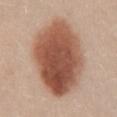Findings:
– follow-up: no biopsy performed (imaged during a skin exam)
– diameter: ~10 mm (longest diameter)
– image-analysis metrics: a symmetry-axis asymmetry near 0.1; a lesion–skin lightness drop of about 17 and a normalized lesion–skin contrast near 11
– body site: the lower back
– acquisition: ~15 mm crop, total-body skin-cancer survey
– subject: female, roughly 20 years of age